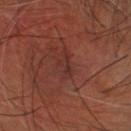Q: Was this lesion biopsied?
A: catalogued during a skin exam; not biopsied